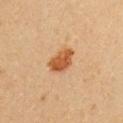Case summary:
• site · the front of the torso
• subject · female, aged approximately 20
• lighting · cross-polarized illumination
• image source · total-body-photography crop, ~15 mm field of view